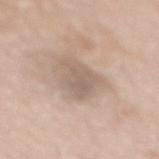Imaged during a routine full-body skin examination; the lesion was not biopsied and no histopathology is available.
The lesion-visualizer software estimated an area of roughly 8 mm², an eccentricity of roughly 0.75, and a shape-asymmetry score of about 0.3 (0 = symmetric). The analysis additionally found a lesion color around L≈60 a*≈13 b*≈25 in CIELAB and a lesion–skin lightness drop of about 9. It also reported a classifier nevus-likeness of about 0/100 and a detector confidence of about 85 out of 100 that the crop contains a lesion.
A female subject aged approximately 60.
A lesion tile, about 15 mm wide, cut from a 3D total-body photograph.
On the mid back.